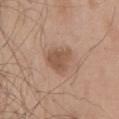biopsy status: imaged on a skin check; not biopsied | anatomic site: the chest | lighting: white-light illumination | patient: male, in their mid- to late 60s | diameter: about 3.5 mm | automated metrics: a footprint of about 7 mm², an outline eccentricity of about 0.6 (0 = round, 1 = elongated), and a symmetry-axis asymmetry near 0.35; a lesion color around L≈53 a*≈18 b*≈29 in CIELAB and about 10 CIELAB-L* units darker than the surrounding skin; a classifier nevus-likeness of about 15/100 and a lesion-detection confidence of about 100/100 | image: ~15 mm crop, total-body skin-cancer survey.